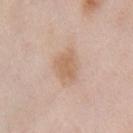A 15 mm close-up extracted from a 3D total-body photography capture.
The lesion is located on the chest.
A male patient, in their mid- to late 50s.